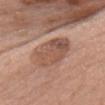notes: no biopsy performed (imaged during a skin exam) | lighting: white-light | size: ~5 mm (longest diameter) | subject: female, aged approximately 60 | site: the head or neck | image-analysis metrics: a border-irregularity index near 1.5/10, a color-variation rating of about 5.5/10, and radial color variation of about 2; a classifier nevus-likeness of about 75/100 and a lesion-detection confidence of about 95/100 | imaging modality: 15 mm crop, total-body photography.